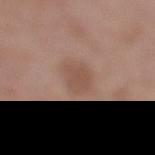{"lesion_size": {"long_diameter_mm_approx": 4.0}, "image": {"source": "total-body photography crop", "field_of_view_mm": 15}, "site": "left lower leg", "patient": {"sex": "female", "age_approx": 55}, "lighting": "white-light"}